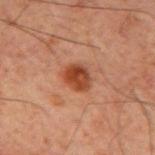Findings:
- biopsy status: imaged on a skin check; not biopsied
- location: the back
- size: about 3.5 mm
- subject: male, aged approximately 60
- image source: ~15 mm tile from a whole-body skin photo
- tile lighting: cross-polarized
- TBP lesion metrics: a mean CIELAB color near L≈34 a*≈23 b*≈28, about 10 CIELAB-L* units darker than the surrounding skin, and a normalized lesion–skin contrast near 9.5; an automated nevus-likeness rating near 95 out of 100 and lesion-presence confidence of about 100/100